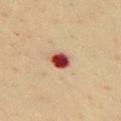No biopsy was performed on this lesion — it was imaged during a full skin examination and was not determined to be concerning. Approximately 3 mm at its widest. Captured under cross-polarized illumination. The total-body-photography lesion software estimated a border-irregularity index near 1.5/10, internal color variation of about 8 on a 0–10 scale, and a peripheral color-asymmetry measure near 2.5. And it measured a nevus-likeness score of about 0/100. A female patient, aged around 60. From the chest. This image is a 15 mm lesion crop taken from a total-body photograph.